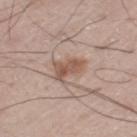Clinical impression: No biopsy was performed on this lesion — it was imaged during a full skin examination and was not determined to be concerning. Context: Located on the right leg. Measured at roughly 4 mm in maximum diameter. A male patient roughly 70 years of age. A 15 mm close-up extracted from a 3D total-body photography capture. The tile uses white-light illumination. The lesion-visualizer software estimated a footprint of about 6 mm² and an outline eccentricity of about 0.85 (0 = round, 1 = elongated). And it measured a lesion color around L≈54 a*≈18 b*≈27 in CIELAB and a lesion–skin lightness drop of about 10. The software also gave border irregularity of about 4 on a 0–10 scale, a within-lesion color-variation index near 3/10, and peripheral color asymmetry of about 1.